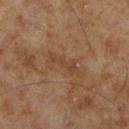Clinical impression: Recorded during total-body skin imaging; not selected for excision or biopsy. Image and clinical context: The lesion is located on the right lower leg. The subject is a male roughly 70 years of age. The tile uses cross-polarized illumination. A close-up tile cropped from a whole-body skin photograph, about 15 mm across. Longest diameter approximately 4.5 mm.